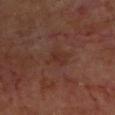follow-up — imaged on a skin check; not biopsied | lighting — cross-polarized | anatomic site — the upper back | image-analysis metrics — a footprint of about 5 mm², an eccentricity of roughly 0.75, and a shape-asymmetry score of about 0.45 (0 = symmetric); a mean CIELAB color near L≈30 a*≈19 b*≈24, a lesion–skin lightness drop of about 4, and a normalized lesion–skin contrast near 5; border irregularity of about 4.5 on a 0–10 scale and a within-lesion color-variation index near 2/10 | patient — male, in their 60s | image source — ~15 mm crop, total-body skin-cancer survey.